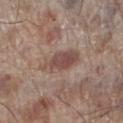Q: Is there a histopathology result?
A: total-body-photography surveillance lesion; no biopsy
Q: What are the patient's age and sex?
A: male, in their 70s
Q: What kind of image is this?
A: ~15 mm tile from a whole-body skin photo
Q: Lesion location?
A: the left lower leg